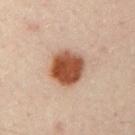Recorded during total-body skin imaging; not selected for excision or biopsy. Located on the arm. A 15 mm close-up extracted from a 3D total-body photography capture. A male patient about 50 years old. Imaged with cross-polarized lighting.